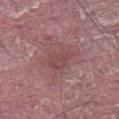Q: Is there a histopathology result?
A: no biopsy performed (imaged during a skin exam)
Q: Lesion location?
A: the right thigh
Q: What are the patient's age and sex?
A: male, about 40 years old
Q: How was this image acquired?
A: ~15 mm tile from a whole-body skin photo
Q: How large is the lesion?
A: ~3.5 mm (longest diameter)